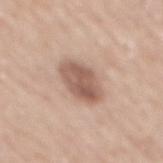Part of a total-body skin-imaging series; this lesion was reviewed on a skin check and was not flagged for biopsy. Captured under white-light illumination. The lesion's longest dimension is about 5 mm. A male patient aged around 70. Cropped from a total-body skin-imaging series; the visible field is about 15 mm. Located on the mid back.